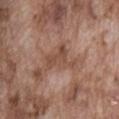{"image": {"source": "total-body photography crop", "field_of_view_mm": 15}, "site": "abdomen", "lighting": "white-light", "patient": {"sex": "male", "age_approx": 75}, "lesion_size": {"long_diameter_mm_approx": 4.5}}